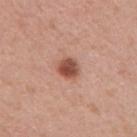Captured during whole-body skin photography for melanoma surveillance; the lesion was not biopsied.
From the right upper arm.
A female subject aged around 40.
Cropped from a whole-body photographic skin survey; the tile spans about 15 mm.
The recorded lesion diameter is about 2.5 mm.
The tile uses white-light illumination.